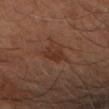Captured during whole-body skin photography for melanoma surveillance; the lesion was not biopsied.
A close-up tile cropped from a whole-body skin photograph, about 15 mm across.
A male patient aged around 70.
Imaged with cross-polarized lighting.
The lesion is located on the left forearm.
About 2.5 mm across.